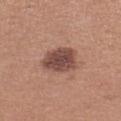follow-up: imaged on a skin check; not biopsied | lesion size: ~4 mm (longest diameter) | site: the left lower leg | lighting: white-light | image-analysis metrics: a footprint of about 12 mm², an outline eccentricity of about 0.45 (0 = round, 1 = elongated), and a shape-asymmetry score of about 0.25 (0 = symmetric); a lesion color around L≈47 a*≈21 b*≈24 in CIELAB, a lesion–skin lightness drop of about 14, and a normalized lesion–skin contrast near 10; border irregularity of about 2 on a 0–10 scale, internal color variation of about 3.5 on a 0–10 scale, and radial color variation of about 1; a classifier nevus-likeness of about 35/100 and lesion-presence confidence of about 100/100 | image: 15 mm crop, total-body photography | patient: female, aged around 40.